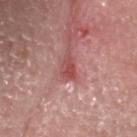lesion diameter: ≈3 mm | patient: male, aged approximately 75 | body site: the head or neck | imaging modality: ~15 mm tile from a whole-body skin photo | illumination: white-light illumination.